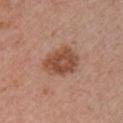Clinical summary:
Located on the arm. Approximately 4.5 mm at its widest. Captured under white-light illumination. A female patient in their mid-50s. A close-up tile cropped from a whole-body skin photograph, about 15 mm across.